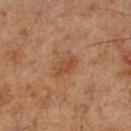| field | value |
|---|---|
| acquisition | ~15 mm crop, total-body skin-cancer survey |
| body site | the left lower leg |
| tile lighting | cross-polarized |
| patient | male, aged around 70 |